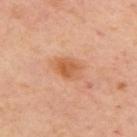| feature | finding |
|---|---|
| biopsy status | total-body-photography surveillance lesion; no biopsy |
| body site | the arm |
| imaging modality | ~15 mm crop, total-body skin-cancer survey |
| subject | female, roughly 40 years of age |
| size | ≈3.5 mm |
| tile lighting | cross-polarized illumination |
| automated metrics | an area of roughly 7 mm², an outline eccentricity of about 0.7 (0 = round, 1 = elongated), and a shape-asymmetry score of about 0.2 (0 = symmetric); a border-irregularity index near 2/10, a within-lesion color-variation index near 4/10, and radial color variation of about 1.5; a lesion-detection confidence of about 100/100 |